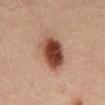No biopsy was performed on this lesion — it was imaged during a full skin examination and was not determined to be concerning.
A male patient aged 48 to 52.
This is a cross-polarized tile.
On the abdomen.
Automated tile analysis of the lesion measured a border-irregularity index near 1.5/10, a within-lesion color-variation index near 5.5/10, and a peripheral color-asymmetry measure near 1.5.
Cropped from a whole-body photographic skin survey; the tile spans about 15 mm.